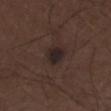biopsy status: imaged on a skin check; not biopsied | subject: male, aged around 50 | diameter: about 3 mm | lighting: white-light | image: total-body-photography crop, ~15 mm field of view | anatomic site: the left thigh.